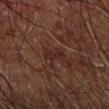Captured during whole-body skin photography for melanoma surveillance; the lesion was not biopsied. A male patient, about 70 years old. Located on the right forearm. About 3 mm across. A 15 mm crop from a total-body photograph taken for skin-cancer surveillance. This is a cross-polarized tile.